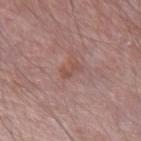follow-up — total-body-photography surveillance lesion; no biopsy | subject — male, about 65 years old | location — the left forearm | image source — ~15 mm tile from a whole-body skin photo.